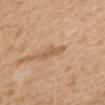<tbp_lesion>
<site>mid back</site>
<lesion_size>
  <long_diameter_mm_approx>3.5</long_diameter_mm_approx>
</lesion_size>
<patient>
  <sex>female</sex>
  <age_approx>70</age_approx>
</patient>
<image>
  <source>total-body photography crop</source>
  <field_of_view_mm>15</field_of_view_mm>
</image>
<automated_metrics>
  <border_irregularity_0_10>5.5</border_irregularity_0_10>
  <color_variation_0_10>0.0</color_variation_0_10>
  <peripheral_color_asymmetry>0.0</peripheral_color_asymmetry>
</automated_metrics>
<lighting>white-light</lighting>
</tbp_lesion>